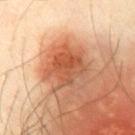Part of a total-body skin-imaging series; this lesion was reviewed on a skin check and was not flagged for biopsy.
About 4.5 mm across.
The subject is a male aged 38 to 42.
From the chest.
A 15 mm crop from a total-body photograph taken for skin-cancer surveillance.
An algorithmic analysis of the crop reported a mean CIELAB color near L≈56 a*≈29 b*≈38, a lesion–skin lightness drop of about 12, and a normalized lesion–skin contrast near 8. And it measured border irregularity of about 4 on a 0–10 scale and internal color variation of about 5.5 on a 0–10 scale.
Imaged with cross-polarized lighting.